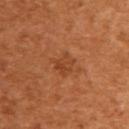biopsy_status: not biopsied; imaged during a skin examination
patient:
  sex: female
  age_approx: 55
lesion_size:
  long_diameter_mm_approx: 3.0
image:
  source: total-body photography crop
  field_of_view_mm: 15
site: upper back